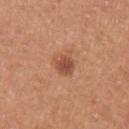  biopsy_status: not biopsied; imaged during a skin examination
  lesion_size:
    long_diameter_mm_approx: 2.5
  site: arm
  lighting: white-light
  patient:
    sex: male
    age_approx: 30
  image:
    source: total-body photography crop
    field_of_view_mm: 15
  automated_metrics:
    area_mm2_approx: 5.0
    eccentricity: 0.35
    shape_asymmetry: 0.2
    nevus_likeness_0_100: 85
    lesion_detection_confidence_0_100: 100